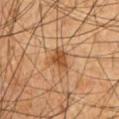Approximately 3 mm at its widest.
A male subject in their mid- to late 60s.
The total-body-photography lesion software estimated a normalized lesion–skin contrast near 8.5. And it measured a border-irregularity rating of about 3.5/10, a color-variation rating of about 2/10, and a peripheral color-asymmetry measure near 0.5.
From the front of the torso.
Captured under cross-polarized illumination.
Cropped from a whole-body photographic skin survey; the tile spans about 15 mm.